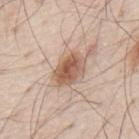Imaged during a routine full-body skin examination; the lesion was not biopsied and no histopathology is available.
The recorded lesion diameter is about 4.5 mm.
This is a white-light tile.
A region of skin cropped from a whole-body photographic capture, roughly 15 mm wide.
Located on the mid back.
A male subject, approximately 80 years of age.
An algorithmic analysis of the crop reported a footprint of about 9.5 mm² and a shape eccentricity near 0.75. The analysis additionally found a mean CIELAB color near L≈58 a*≈19 b*≈29, a lesion–skin lightness drop of about 13, and a normalized border contrast of about 9. And it measured a border-irregularity index near 2/10, a within-lesion color-variation index near 6/10, and radial color variation of about 2.